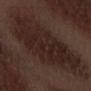subject = male, aged 68–72
anatomic site = the left forearm
tile lighting = white-light illumination
image = ~15 mm crop, total-body skin-cancer survey
diameter = ~11.5 mm (longest diameter)